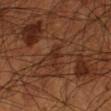biopsy status: no biopsy performed (imaged during a skin exam)
TBP lesion metrics: an eccentricity of roughly 0.8 and a symmetry-axis asymmetry near 0.25; a lesion–skin lightness drop of about 5 and a normalized border contrast of about 6; border irregularity of about 3 on a 0–10 scale, internal color variation of about 1.5 on a 0–10 scale, and a peripheral color-asymmetry measure near 0.5
body site: the right forearm
size: ≈2.5 mm
subject: male, roughly 65 years of age
image source: ~15 mm tile from a whole-body skin photo